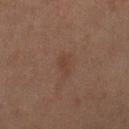Part of a total-body skin-imaging series; this lesion was reviewed on a skin check and was not flagged for biopsy.
The recorded lesion diameter is about 3 mm.
Cropped from a total-body skin-imaging series; the visible field is about 15 mm.
The patient is a female aged approximately 55.
Automated image analysis of the tile measured an area of roughly 3.5 mm², an eccentricity of roughly 0.85, and a symmetry-axis asymmetry near 0.35. It also reported a lesion-to-skin contrast of about 4.5 (normalized; higher = more distinct). And it measured an automated nevus-likeness rating near 0 out of 100.
From the right thigh.